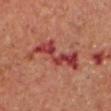Clinical impression: No biopsy was performed on this lesion — it was imaged during a full skin examination and was not determined to be concerning. Background: The patient is a male aged around 70. A region of skin cropped from a whole-body photographic capture, roughly 15 mm wide. The lesion is on the head or neck.